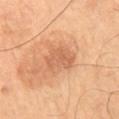notes = total-body-photography surveillance lesion; no biopsy
patient = male, aged approximately 65
image source = 15 mm crop, total-body photography
illumination = cross-polarized
location = the right thigh
size = about 4 mm